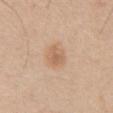Assessment: The lesion was tiled from a total-body skin photograph and was not biopsied. Image and clinical context: Automated tile analysis of the lesion measured a footprint of about 6.5 mm² and a shape eccentricity near 0.4. The software also gave a mean CIELAB color near L≈62 a*≈19 b*≈33. Captured under white-light illumination. A male subject, in their mid-60s. A 15 mm close-up tile from a total-body photography series done for melanoma screening. The lesion is located on the abdomen. Longest diameter approximately 3 mm.